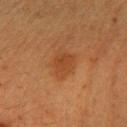Clinical impression: Captured during whole-body skin photography for melanoma surveillance; the lesion was not biopsied. Clinical summary: A female patient about 40 years old. About 4 mm across. A 15 mm close-up extracted from a 3D total-body photography capture. An algorithmic analysis of the crop reported a lesion area of about 8 mm² and a symmetry-axis asymmetry near 0.25. The software also gave border irregularity of about 2.5 on a 0–10 scale, internal color variation of about 2 on a 0–10 scale, and radial color variation of about 0.5. And it measured lesion-presence confidence of about 100/100. Located on the right forearm.